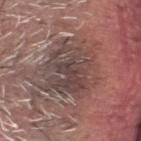This lesion was catalogued during total-body skin photography and was not selected for biopsy. On the head or neck. The subject is a male aged around 60. A lesion tile, about 15 mm wide, cut from a 3D total-body photograph.